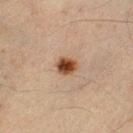This lesion was catalogued during total-body skin photography and was not selected for biopsy.
A 15 mm close-up extracted from a 3D total-body photography capture.
Imaged with cross-polarized lighting.
A male subject aged approximately 45.
The lesion is located on the left lower leg.
About 2.5 mm across.